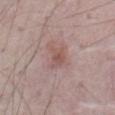follow-up: no biopsy performed (imaged during a skin exam)
site: the abdomen
acquisition: ~15 mm crop, total-body skin-cancer survey
subject: male, roughly 75 years of age
illumination: white-light
lesion diameter: about 3 mm